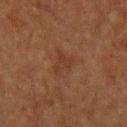No biopsy was performed on this lesion — it was imaged during a full skin examination and was not determined to be concerning. A male subject roughly 50 years of age. The lesion is on the upper back. About 2.5 mm across. The tile uses cross-polarized illumination. A lesion tile, about 15 mm wide, cut from a 3D total-body photograph.